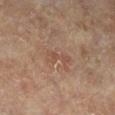The lesion was photographed on a routine skin check and not biopsied; there is no pathology result.
A male patient, about 85 years old or older.
Captured under cross-polarized illumination.
Approximately 3.5 mm at its widest.
From the leg.
A 15 mm crop from a total-body photograph taken for skin-cancer surveillance.